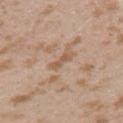| key | value |
|---|---|
| follow-up | no biopsy performed (imaged during a skin exam) |
| body site | the left upper arm |
| patient | female, in their mid-20s |
| imaging modality | 15 mm crop, total-body photography |